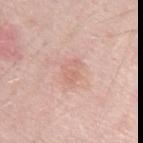Clinical impression: The lesion was photographed on a routine skin check and not biopsied; there is no pathology result. Background: Imaged with white-light lighting. The subject is a male aged 48 to 52. Longest diameter approximately 3 mm. Located on the left upper arm. A region of skin cropped from a whole-body photographic capture, roughly 15 mm wide.